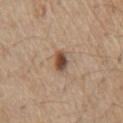No biopsy was performed on this lesion — it was imaged during a full skin examination and was not determined to be concerning.
A close-up tile cropped from a whole-body skin photograph, about 15 mm across.
The subject is a male about 70 years old.
About 2.5 mm across.
The tile uses white-light illumination.
Located on the chest.